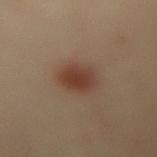biopsy_status: not biopsied; imaged during a skin examination
image:
  source: total-body photography crop
  field_of_view_mm: 15
patient:
  sex: female
  age_approx: 60
site: mid back
lesion_size:
  long_diameter_mm_approx: 4.5
lighting: cross-polarized